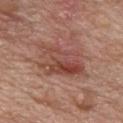Clinical impression:
Imaged during a routine full-body skin examination; the lesion was not biopsied and no histopathology is available.
Clinical summary:
A male subject, aged approximately 70. From the chest. A close-up tile cropped from a whole-body skin photograph, about 15 mm across. About 6.5 mm across.